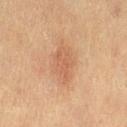A roughly 15 mm field-of-view crop from a total-body skin photograph. The lesion is on the leg. This is a cross-polarized tile. The patient is a female aged approximately 55. The lesion-visualizer software estimated a lesion area of about 10 mm² and an outline eccentricity of about 0.7 (0 = round, 1 = elongated). It also reported a border-irregularity index near 2.5/10, a within-lesion color-variation index near 2/10, and a peripheral color-asymmetry measure near 1. The analysis additionally found a lesion-detection confidence of about 100/100. Measured at roughly 4.5 mm in maximum diameter.